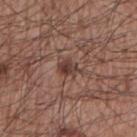Q: Is there a histopathology result?
A: imaged on a skin check; not biopsied
Q: Lesion size?
A: about 2.5 mm
Q: What is the anatomic site?
A: the right upper arm
Q: Who is the patient?
A: male, in their mid- to late 60s
Q: Automated lesion metrics?
A: a mean CIELAB color near L≈39 a*≈19 b*≈22, a lesion–skin lightness drop of about 10, and a normalized border contrast of about 8.5; a color-variation rating of about 4/10 and a peripheral color-asymmetry measure near 1.5; a detector confidence of about 100 out of 100 that the crop contains a lesion
Q: How was this image acquired?
A: ~15 mm crop, total-body skin-cancer survey